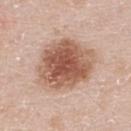Part of a total-body skin-imaging series; this lesion was reviewed on a skin check and was not flagged for biopsy. The subject is a male in their 40s. Cropped from a whole-body photographic skin survey; the tile spans about 15 mm. The recorded lesion diameter is about 7.5 mm. Automated image analysis of the tile measured a lesion area of about 33 mm², an eccentricity of roughly 0.6, and a shape-asymmetry score of about 0.2 (0 = symmetric). The analysis additionally found a lesion–skin lightness drop of about 15. The software also gave peripheral color asymmetry of about 1.5. And it measured a classifier nevus-likeness of about 95/100 and a lesion-detection confidence of about 100/100. From the upper back.